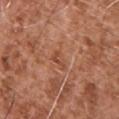follow-up — catalogued during a skin exam; not biopsied
image source — total-body-photography crop, ~15 mm field of view
patient — male, aged approximately 75
body site — the upper back